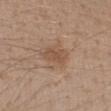Assessment: This lesion was catalogued during total-body skin photography and was not selected for biopsy. Context: Imaged with white-light lighting. Approximately 3 mm at its widest. A female subject aged 28 to 32. The lesion is located on the left forearm. The total-body-photography lesion software estimated an outline eccentricity of about 0.55 (0 = round, 1 = elongated) and a symmetry-axis asymmetry near 0.2. And it measured a lesion color around L≈51 a*≈18 b*≈30 in CIELAB, about 7 CIELAB-L* units darker than the surrounding skin, and a lesion-to-skin contrast of about 6 (normalized; higher = more distinct). The analysis additionally found a border-irregularity index near 2.5/10, internal color variation of about 1.5 on a 0–10 scale, and radial color variation of about 0.5. And it measured an automated nevus-likeness rating near 25 out of 100 and a detector confidence of about 100 out of 100 that the crop contains a lesion. A close-up tile cropped from a whole-body skin photograph, about 15 mm across.